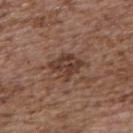Clinical impression:
The lesion was photographed on a routine skin check and not biopsied; there is no pathology result.
Clinical summary:
A female patient, approximately 65 years of age. About 4 mm across. From the back. This is a white-light tile. This image is a 15 mm lesion crop taken from a total-body photograph.